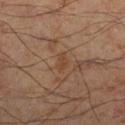Imaged during a routine full-body skin examination; the lesion was not biopsied and no histopathology is available.
The patient is a male about 60 years old.
Captured under cross-polarized illumination.
The recorded lesion diameter is about 2.5 mm.
Automated tile analysis of the lesion measured an average lesion color of about L≈38 a*≈17 b*≈28 (CIELAB), about 5 CIELAB-L* units darker than the surrounding skin, and a normalized lesion–skin contrast near 5.5. And it measured border irregularity of about 2.5 on a 0–10 scale, a color-variation rating of about 0.5/10, and a peripheral color-asymmetry measure near 0. And it measured a classifier nevus-likeness of about 0/100.
A 15 mm close-up tile from a total-body photography series done for melanoma screening.
On the leg.